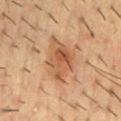Notes:
– biopsy status · imaged on a skin check; not biopsied
– subject · male, roughly 55 years of age
– body site · the mid back
– tile lighting · cross-polarized
– diameter · ≈5.5 mm
– image source · total-body-photography crop, ~15 mm field of view